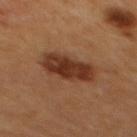notes = imaged on a skin check; not biopsied | subject = male | location = the mid back | lighting = cross-polarized | image = total-body-photography crop, ~15 mm field of view | size = ≈5.5 mm.